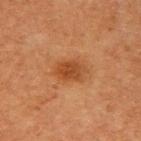The lesion was photographed on a routine skin check and not biopsied; there is no pathology result. A 15 mm close-up tile from a total-body photography series done for melanoma screening. On the right upper arm. Measured at roughly 4 mm in maximum diameter. A male patient about 75 years old.